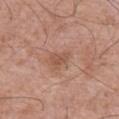| feature | finding |
|---|---|
| notes | imaged on a skin check; not biopsied |
| TBP lesion metrics | a footprint of about 4 mm², an eccentricity of roughly 0.55, and a shape-asymmetry score of about 0.35 (0 = symmetric); a normalized lesion–skin contrast near 5.5; a border-irregularity rating of about 3.5/10 and a color-variation rating of about 2.5/10; a classifier nevus-likeness of about 0/100 and lesion-presence confidence of about 100/100 |
| lesion size | about 2.5 mm |
| anatomic site | the abdomen |
| patient | male, aged 68–72 |
| tile lighting | white-light |
| image | ~15 mm tile from a whole-body skin photo |